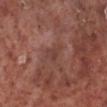Q: Was a biopsy performed?
A: imaged on a skin check; not biopsied
Q: Where on the body is the lesion?
A: the left lower leg
Q: How was this image acquired?
A: 15 mm crop, total-body photography
Q: Patient demographics?
A: male, aged 53 to 57